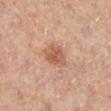Impression: The lesion was tiled from a total-body skin photograph and was not biopsied. Context: The lesion is on the leg. A region of skin cropped from a whole-body photographic capture, roughly 15 mm wide. A female subject aged around 45. Captured under cross-polarized illumination.